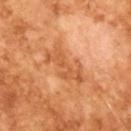Case summary:
– biopsy status — no biopsy performed (imaged during a skin exam)
– illumination — cross-polarized
– patient — male, aged approximately 65
– size — about 5.5 mm
– imaging modality — 15 mm crop, total-body photography